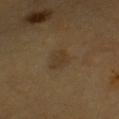The lesion was tiled from a total-body skin photograph and was not biopsied.
This image is a 15 mm lesion crop taken from a total-body photograph.
Located on the chest.
A male subject, aged 58 to 62.